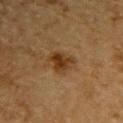Impression: Captured during whole-body skin photography for melanoma surveillance; the lesion was not biopsied. Acquisition and patient details: About 3.5 mm across. A male subject approximately 85 years of age or older. A region of skin cropped from a whole-body photographic capture, roughly 15 mm wide. The tile uses cross-polarized illumination. An algorithmic analysis of the crop reported a mean CIELAB color near L≈31 a*≈17 b*≈31, about 9 CIELAB-L* units darker than the surrounding skin, and a normalized lesion–skin contrast near 9.5. It also reported a classifier nevus-likeness of about 90/100 and a detector confidence of about 100 out of 100 that the crop contains a lesion. The lesion is on the upper back.